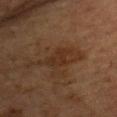Impression:
Captured during whole-body skin photography for melanoma surveillance; the lesion was not biopsied.
Background:
About 4 mm across. The total-body-photography lesion software estimated a lesion area of about 6 mm², a shape eccentricity near 0.8, and a shape-asymmetry score of about 0.35 (0 = symmetric). And it measured an automated nevus-likeness rating near 0 out of 100 and lesion-presence confidence of about 95/100. From the chest. Cropped from a whole-body photographic skin survey; the tile spans about 15 mm. Imaged with cross-polarized lighting. A female subject, aged approximately 60.